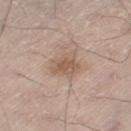biopsy status — no biopsy performed (imaged during a skin exam)
image — ~15 mm tile from a whole-body skin photo
anatomic site — the right thigh
tile lighting — white-light
lesion size — ~3.5 mm (longest diameter)
subject — male, aged 68 to 72